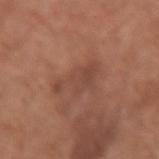Q: Is there a histopathology result?
A: no biopsy performed (imaged during a skin exam)